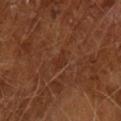Q: Was this lesion biopsied?
A: total-body-photography surveillance lesion; no biopsy
Q: What are the patient's age and sex?
A: male, aged approximately 65
Q: What kind of image is this?
A: ~15 mm crop, total-body skin-cancer survey